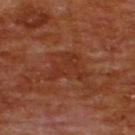Imaged during a routine full-body skin examination; the lesion was not biopsied and no histopathology is available.
A male patient, in their mid-60s.
A roughly 15 mm field-of-view crop from a total-body skin photograph.
Measured at roughly 4.5 mm in maximum diameter.
The lesion-visualizer software estimated a mean CIELAB color near L≈31 a*≈25 b*≈30, about 5 CIELAB-L* units darker than the surrounding skin, and a normalized lesion–skin contrast near 5. The analysis additionally found a border-irregularity index near 7.5/10, internal color variation of about 2 on a 0–10 scale, and a peripheral color-asymmetry measure near 0.5. And it measured a nevus-likeness score of about 0/100 and lesion-presence confidence of about 100/100.
The tile uses cross-polarized illumination.
Located on the upper back.